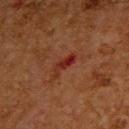{
  "biopsy_status": "not biopsied; imaged during a skin examination",
  "automated_metrics": {
    "eccentricity": 0.95,
    "shape_asymmetry": 0.35,
    "cielab_L": 24,
    "cielab_a": 26,
    "cielab_b": 26,
    "vs_skin_contrast_norm": 8.0,
    "border_irregularity_0_10": 4.5,
    "color_variation_0_10": 0.0,
    "peripheral_color_asymmetry": 0.0,
    "nevus_likeness_0_100": 0,
    "lesion_detection_confidence_0_100": 100
  },
  "lesion_size": {
    "long_diameter_mm_approx": 3.5
  },
  "patient": {
    "sex": "male",
    "age_approx": 65
  },
  "site": "back",
  "image": {
    "source": "total-body photography crop",
    "field_of_view_mm": 15
  }
}